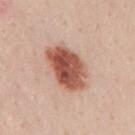Clinical impression: This lesion was catalogued during total-body skin photography and was not selected for biopsy. Image and clinical context: This is a white-light tile. The subject is a male about 50 years old. Located on the mid back. Approximately 6.5 mm at its widest. A region of skin cropped from a whole-body photographic capture, roughly 15 mm wide. Automated tile analysis of the lesion measured a lesion area of about 18 mm² and a shape eccentricity near 0.85. And it measured an average lesion color of about L≈55 a*≈25 b*≈29 (CIELAB), a lesion–skin lightness drop of about 17, and a lesion-to-skin contrast of about 11 (normalized; higher = more distinct). The analysis additionally found a border-irregularity index near 2/10 and a within-lesion color-variation index near 6.5/10. The analysis additionally found a lesion-detection confidence of about 100/100.